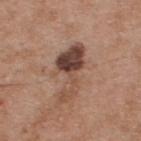size = ~6.5 mm (longest diameter) | image-analysis metrics = a footprint of about 13 mm²; an average lesion color of about L≈45 a*≈19 b*≈26 (CIELAB) and roughly 14 lightness units darker than nearby skin; a nevus-likeness score of about 20/100 and a detector confidence of about 100 out of 100 that the crop contains a lesion | subject = male, aged around 55 | tile lighting = white-light illumination | image = total-body-photography crop, ~15 mm field of view | site = the upper back.